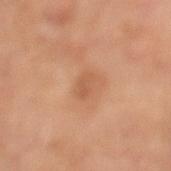Recorded during total-body skin imaging; not selected for excision or biopsy.
Imaged with cross-polarized lighting.
Located on the leg.
A female patient, aged approximately 65.
About 2.5 mm across.
Cropped from a whole-body photographic skin survey; the tile spans about 15 mm.